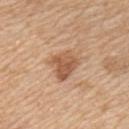The lesion was tiled from a total-body skin photograph and was not biopsied. The lesion is located on the left upper arm. Automated tile analysis of the lesion measured a border-irregularity rating of about 3/10, internal color variation of about 4 on a 0–10 scale, and radial color variation of about 1.5. A close-up tile cropped from a whole-body skin photograph, about 15 mm across. A male subject, aged 58–62.